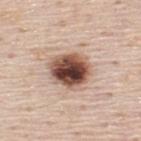Findings:
- workup: total-body-photography surveillance lesion; no biopsy
- automated lesion analysis: a mean CIELAB color near L≈50 a*≈20 b*≈26, roughly 22 lightness units darker than nearby skin, and a normalized lesion–skin contrast near 14
- imaging modality: ~15 mm crop, total-body skin-cancer survey
- patient: male, in their mid- to late 40s
- location: the back
- diameter: ≈5 mm
- lighting: white-light